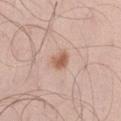Impression:
The lesion was tiled from a total-body skin photograph and was not biopsied.